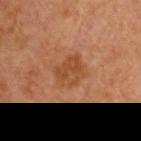biopsy status — no biopsy performed (imaged during a skin exam) | image source — ~15 mm crop, total-body skin-cancer survey | site — the right upper arm | subject — male, aged approximately 65 | diameter — about 3.5 mm | illumination — cross-polarized.